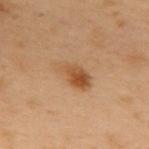– biopsy status — catalogued during a skin exam; not biopsied
– image source — ~15 mm crop, total-body skin-cancer survey
– lesion diameter — ~4.5 mm (longest diameter)
– patient — male, approximately 55 years of age
– lighting — cross-polarized illumination
– anatomic site — the mid back
– TBP lesion metrics — a lesion area of about 9 mm² and two-axis asymmetry of about 0.2; a mean CIELAB color near L≈42 a*≈17 b*≈31, a lesion–skin lightness drop of about 8, and a normalized border contrast of about 7; a within-lesion color-variation index near 6.5/10 and peripheral color asymmetry of about 2.5